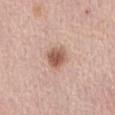The lesion was photographed on a routine skin check and not biopsied; there is no pathology result. This is a white-light tile. Measured at roughly 3 mm in maximum diameter. A female patient, in their mid- to late 60s. Automated tile analysis of the lesion measured a lesion area of about 6.5 mm², an outline eccentricity of about 0.5 (0 = round, 1 = elongated), and a symmetry-axis asymmetry near 0.25. It also reported a lesion color around L≈57 a*≈21 b*≈29 in CIELAB, roughly 13 lightness units darker than nearby skin, and a normalized lesion–skin contrast near 8.5. And it measured border irregularity of about 2 on a 0–10 scale and a color-variation rating of about 4.5/10. The software also gave a classifier nevus-likeness of about 95/100. Located on the abdomen. Cropped from a total-body skin-imaging series; the visible field is about 15 mm.